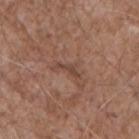<case>
<biopsy_status>not biopsied; imaged during a skin examination</biopsy_status>
<image>
  <source>total-body photography crop</source>
  <field_of_view_mm>15</field_of_view_mm>
</image>
<site>chest</site>
<patient>
  <sex>male</sex>
  <age_approx>70</age_approx>
</patient>
</case>